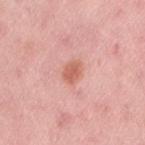Q: Is there a histopathology result?
A: imaged on a skin check; not biopsied
Q: Automated lesion metrics?
A: a nevus-likeness score of about 80/100 and a detector confidence of about 100 out of 100 that the crop contains a lesion
Q: What are the patient's age and sex?
A: female, aged 48 to 52
Q: How was the tile lit?
A: white-light
Q: What is the anatomic site?
A: the lower back
Q: What is the imaging modality?
A: 15 mm crop, total-body photography
Q: What is the lesion's diameter?
A: about 2.5 mm